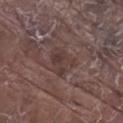<lesion>
  <biopsy_status>not biopsied; imaged during a skin examination</biopsy_status>
  <image>
    <source>total-body photography crop</source>
    <field_of_view_mm>15</field_of_view_mm>
  </image>
  <site>right lower leg</site>
  <patient>
    <sex>male</sex>
    <age_approx>80</age_approx>
  </patient>
  <lighting>white-light</lighting>
  <automated_metrics>
    <cielab_L>37</cielab_L>
    <cielab_a>16</cielab_a>
    <cielab_b>18</cielab_b>
    <vs_skin_darker_L>6.0</vs_skin_darker_L>
    <vs_skin_contrast_norm>5.5</vs_skin_contrast_norm>
    <border_irregularity_0_10>4.0</border_irregularity_0_10>
    <color_variation_0_10>3.5</color_variation_0_10>
  </automated_metrics>
  <lesion_size>
    <long_diameter_mm_approx>3.5</long_diameter_mm_approx>
  </lesion_size>
</lesion>